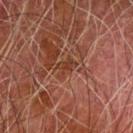- notes: no biopsy performed (imaged during a skin exam)
- size: ≈2.5 mm
- tile lighting: cross-polarized illumination
- imaging modality: 15 mm crop, total-body photography
- subject: male, about 80 years old
- anatomic site: the left forearm
- automated lesion analysis: roughly 6 lightness units darker than nearby skin; border irregularity of about 4.5 on a 0–10 scale, internal color variation of about 1 on a 0–10 scale, and radial color variation of about 0